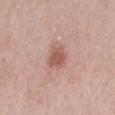No biopsy was performed on this lesion — it was imaged during a full skin examination and was not determined to be concerning. The total-body-photography lesion software estimated an average lesion color of about L≈56 a*≈23 b*≈25 (CIELAB), a lesion–skin lightness drop of about 11, and a normalized border contrast of about 7. The software also gave a classifier nevus-likeness of about 70/100 and a detector confidence of about 100 out of 100 that the crop contains a lesion. This is a white-light tile. On the mid back. Cropped from a whole-body photographic skin survey; the tile spans about 15 mm. The subject is a female aged 48–52.